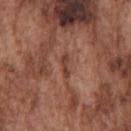biopsy status: total-body-photography surveillance lesion; no biopsy | lighting: white-light | subject: male, in their mid-70s | diameter: ~3 mm (longest diameter) | automated lesion analysis: a footprint of about 2.5 mm², an eccentricity of roughly 0.95, and a shape-asymmetry score of about 0.3 (0 = symmetric); a mean CIELAB color near L≈41 a*≈23 b*≈28, a lesion–skin lightness drop of about 9, and a lesion-to-skin contrast of about 7.5 (normalized; higher = more distinct); a border-irregularity rating of about 4/10 | site: the chest | acquisition: 15 mm crop, total-body photography.